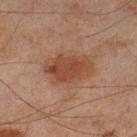workup: imaged on a skin check; not biopsied | automated lesion analysis: a lesion area of about 16 mm², an eccentricity of roughly 0.8, and two-axis asymmetry of about 0.15; a mean CIELAB color near L≈36 a*≈19 b*≈26, a lesion–skin lightness drop of about 8, and a normalized border contrast of about 7.5; a border-irregularity index near 2/10, internal color variation of about 3.5 on a 0–10 scale, and radial color variation of about 1.5; a nevus-likeness score of about 75/100 and a lesion-detection confidence of about 100/100 | lesion diameter: ≈5.5 mm | body site: the left lower leg | patient: male, aged around 45 | acquisition: total-body-photography crop, ~15 mm field of view | illumination: cross-polarized.